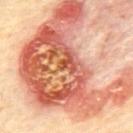| field | value |
|---|---|
| biopsy status | imaged on a skin check; not biopsied |
| tile lighting | cross-polarized |
| patient | male, in their 70s |
| location | the mid back |
| TBP lesion metrics | an outline eccentricity of about 0.65 (0 = round, 1 = elongated) and a symmetry-axis asymmetry near 0.6; a lesion color around L≈63 a*≈30 b*≈35 in CIELAB and roughly 17 lightness units darker than nearby skin; a border-irregularity rating of about 8.5/10, a within-lesion color-variation index near 10/10, and peripheral color asymmetry of about 4.5; a nevus-likeness score of about 0/100 |
| image source | 15 mm crop, total-body photography |